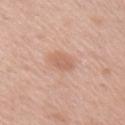Part of a total-body skin-imaging series; this lesion was reviewed on a skin check and was not flagged for biopsy.
A female patient, roughly 40 years of age.
A 15 mm crop from a total-body photograph taken for skin-cancer surveillance.
Automated image analysis of the tile measured border irregularity of about 2 on a 0–10 scale, a color-variation rating of about 1.5/10, and peripheral color asymmetry of about 0.5. The software also gave an automated nevus-likeness rating near 15 out of 100 and a detector confidence of about 100 out of 100 that the crop contains a lesion.
Longest diameter approximately 3 mm.
Captured under white-light illumination.
From the arm.